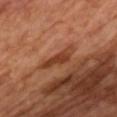The lesion was photographed on a routine skin check and not biopsied; there is no pathology result.
The patient is a male aged around 65.
A 15 mm crop from a total-body photograph taken for skin-cancer surveillance.